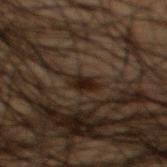Part of a total-body skin-imaging series; this lesion was reviewed on a skin check and was not flagged for biopsy.
The patient is a male roughly 50 years of age.
This is a cross-polarized tile.
A close-up tile cropped from a whole-body skin photograph, about 15 mm across.
On the mid back.
About 2.5 mm across.